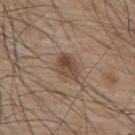Imaged during a routine full-body skin examination; the lesion was not biopsied and no histopathology is available.
A male subject aged 73–77.
The lesion is located on the mid back.
A roughly 15 mm field-of-view crop from a total-body skin photograph.
The tile uses white-light illumination.